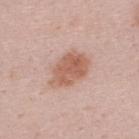Notes:
– biopsy status · imaged on a skin check; not biopsied
– anatomic site · the upper back
– diameter · ≈5 mm
– illumination · white-light
– subject · male, in their mid-20s
– image source · ~15 mm tile from a whole-body skin photo